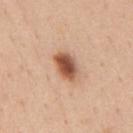{"biopsy_status": "not biopsied; imaged during a skin examination", "automated_metrics": {"area_mm2_approx": 7.0, "eccentricity": 0.75, "border_irregularity_0_10": 1.5, "color_variation_0_10": 6.0, "peripheral_color_asymmetry": 2.0}, "patient": {"sex": "male", "age_approx": 50}, "site": "chest", "image": {"source": "total-body photography crop", "field_of_view_mm": 15}, "lighting": "white-light"}